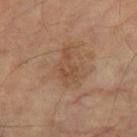Part of a total-body skin-imaging series; this lesion was reviewed on a skin check and was not flagged for biopsy. On the leg. This image is a 15 mm lesion crop taken from a total-body photograph. A male patient, aged around 65.